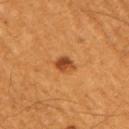Q: Was a biopsy performed?
A: total-body-photography surveillance lesion; no biopsy
Q: Illumination type?
A: cross-polarized
Q: Automated lesion metrics?
A: a lesion color around L≈43 a*≈26 b*≈39 in CIELAB, a lesion–skin lightness drop of about 12, and a lesion-to-skin contrast of about 9.5 (normalized; higher = more distinct); a nevus-likeness score of about 100/100 and a lesion-detection confidence of about 100/100
Q: What kind of image is this?
A: ~15 mm crop, total-body skin-cancer survey
Q: Where on the body is the lesion?
A: the right upper arm
Q: What are the patient's age and sex?
A: male, about 60 years old